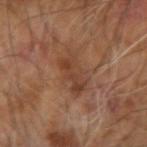follow-up: no biopsy performed (imaged during a skin exam); imaging modality: 15 mm crop, total-body photography; subject: male, aged approximately 60; site: the right arm; lesion diameter: ≈5 mm; lighting: cross-polarized illumination.